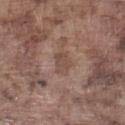patient: male, aged 73 to 77 | location: the left lower leg | size: about 2.5 mm | image source: ~15 mm crop, total-body skin-cancer survey.